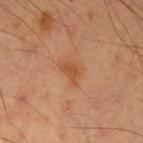Notes:
– notes: no biopsy performed (imaged during a skin exam)
– patient: male, aged 53–57
– image source: total-body-photography crop, ~15 mm field of view
– diameter: about 3 mm
– lighting: cross-polarized illumination
– TBP lesion metrics: a lesion area of about 4 mm², an eccentricity of roughly 0.8, and two-axis asymmetry of about 0.35; a lesion color around L≈43 a*≈22 b*≈33 in CIELAB, a lesion–skin lightness drop of about 7, and a normalized border contrast of about 6.5; a border-irregularity rating of about 3.5/10
– anatomic site: the right upper arm